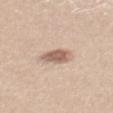biopsy status = no biopsy performed (imaged during a skin exam) | size = about 3 mm | body site = the mid back | subject = female, in their mid- to late 20s | image = total-body-photography crop, ~15 mm field of view.